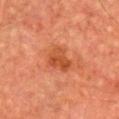<lesion>
  <biopsy_status>not biopsied; imaged during a skin examination</biopsy_status>
  <automated_metrics>
    <eccentricity>0.65</eccentricity>
    <border_irregularity_0_10>3.5</border_irregularity_0_10>
    <color_variation_0_10>3.5</color_variation_0_10>
    <peripheral_color_asymmetry>1.5</peripheral_color_asymmetry>
  </automated_metrics>
  <lighting>cross-polarized</lighting>
  <patient>
    <sex>male</sex>
    <age_approx>65</age_approx>
  </patient>
  <site>chest</site>
  <lesion_size>
    <long_diameter_mm_approx>3.0</long_diameter_mm_approx>
  </lesion_size>
  <image>
    <source>total-body photography crop</source>
    <field_of_view_mm>15</field_of_view_mm>
  </image>
</lesion>